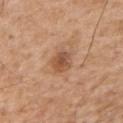Q: Is there a histopathology result?
A: no biopsy performed (imaged during a skin exam)
Q: Who is the patient?
A: male, aged approximately 60
Q: How was this image acquired?
A: total-body-photography crop, ~15 mm field of view
Q: How large is the lesion?
A: about 3 mm
Q: Illumination type?
A: white-light illumination
Q: What did automated image analysis measure?
A: a border-irregularity index near 1.5/10, internal color variation of about 5 on a 0–10 scale, and peripheral color asymmetry of about 1.5; a classifier nevus-likeness of about 30/100 and a lesion-detection confidence of about 100/100
Q: Lesion location?
A: the back